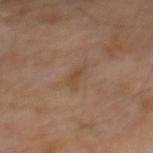Assessment: No biopsy was performed on this lesion — it was imaged during a full skin examination and was not determined to be concerning. Context: The recorded lesion diameter is about 3 mm. This is a cross-polarized tile. A close-up tile cropped from a whole-body skin photograph, about 15 mm across. The subject is a male aged 68 to 72. An algorithmic analysis of the crop reported a classifier nevus-likeness of about 0/100 and a detector confidence of about 100 out of 100 that the crop contains a lesion. The lesion is located on the mid back.